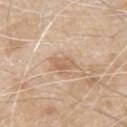follow-up: imaged on a skin check; not biopsied | anatomic site: the left upper arm | tile lighting: white-light illumination | imaging modality: total-body-photography crop, ~15 mm field of view | subject: male, roughly 80 years of age | automated lesion analysis: a footprint of about 4 mm²; a mean CIELAB color near L≈62 a*≈18 b*≈33, roughly 9 lightness units darker than nearby skin, and a lesion-to-skin contrast of about 6 (normalized; higher = more distinct); a border-irregularity rating of about 2/10 and radial color variation of about 1 | size: ~3 mm (longest diameter).